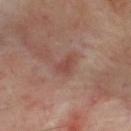Longest diameter approximately 2.5 mm.
A 15 mm close-up tile from a total-body photography series done for melanoma screening.
Located on the upper back.
A male patient, in their 50s.
Captured under cross-polarized illumination.